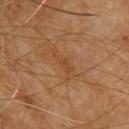No biopsy was performed on this lesion — it was imaged during a full skin examination and was not determined to be concerning.